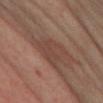The lesion was photographed on a routine skin check and not biopsied; there is no pathology result. A close-up tile cropped from a whole-body skin photograph, about 15 mm across. The lesion is located on the left thigh. A female patient, in their 50s.